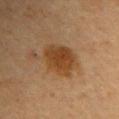Notes:
– notes: no biopsy performed (imaged during a skin exam)
– size: about 5 mm
– anatomic site: the left upper arm
– patient: female, approximately 60 years of age
– lighting: cross-polarized
– image: total-body-photography crop, ~15 mm field of view